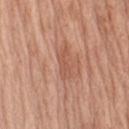Q: How large is the lesion?
A: ~3.5 mm (longest diameter)
Q: Who is the patient?
A: female, aged 73–77
Q: Automated lesion metrics?
A: a footprint of about 3.5 mm², a shape eccentricity near 0.9, and a shape-asymmetry score of about 0.5 (0 = symmetric); a normalized border contrast of about 5.5
Q: What is the anatomic site?
A: the left upper arm
Q: What is the imaging modality?
A: ~15 mm tile from a whole-body skin photo
Q: Illumination type?
A: white-light illumination